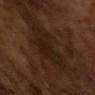Captured during whole-body skin photography for melanoma surveillance; the lesion was not biopsied. A roughly 15 mm field-of-view crop from a total-body skin photograph. The subject is a male in their mid-60s. The lesion's longest dimension is about 5.5 mm.